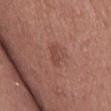Notes:
* biopsy status — total-body-photography surveillance lesion; no biopsy
* body site — the right thigh
* lesion size — ~3 mm (longest diameter)
* subject — female, aged around 40
* image — ~15 mm crop, total-body skin-cancer survey
* tile lighting — white-light illumination